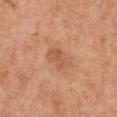Assessment:
The lesion was photographed on a routine skin check and not biopsied; there is no pathology result.
Background:
Imaged with white-light lighting. The total-body-photography lesion software estimated a shape eccentricity near 0.75 and two-axis asymmetry of about 0.2. The software also gave a lesion color around L≈57 a*≈24 b*≈35 in CIELAB, about 7 CIELAB-L* units darker than the surrounding skin, and a lesion-to-skin contrast of about 5 (normalized; higher = more distinct). It also reported a lesion-detection confidence of about 100/100. The lesion's longest dimension is about 3.5 mm. The lesion is on the chest. The subject is a female approximately 55 years of age. A region of skin cropped from a whole-body photographic capture, roughly 15 mm wide.